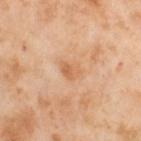Imaged during a routine full-body skin examination; the lesion was not biopsied and no histopathology is available. A female patient aged approximately 55. About 2.5 mm across. Cropped from a total-body skin-imaging series; the visible field is about 15 mm. Located on the left thigh.